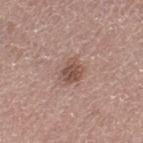* follow-up · catalogued during a skin exam; not biopsied
* site · the left thigh
* image source · 15 mm crop, total-body photography
* TBP lesion metrics · an area of roughly 5.5 mm², a shape eccentricity near 0.6, and a shape-asymmetry score of about 0.3 (0 = symmetric); a mean CIELAB color near L≈50 a*≈20 b*≈25
* subject · female, aged 63 to 67
* tile lighting · white-light illumination
* diameter · about 3 mm